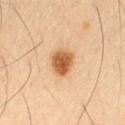This lesion was catalogued during total-body skin photography and was not selected for biopsy. A male subject, roughly 65 years of age. Captured under cross-polarized illumination. A lesion tile, about 15 mm wide, cut from a 3D total-body photograph. The lesion is on the left thigh.